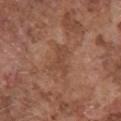Q: Was this lesion biopsied?
A: no biopsy performed (imaged during a skin exam)
Q: How large is the lesion?
A: about 3.5 mm
Q: What kind of image is this?
A: ~15 mm crop, total-body skin-cancer survey
Q: Patient demographics?
A: male, aged 73–77
Q: Where on the body is the lesion?
A: the front of the torso
Q: How was the tile lit?
A: white-light
Q: Automated lesion metrics?
A: a lesion area of about 6 mm², an eccentricity of roughly 0.85, and a shape-asymmetry score of about 0.5 (0 = symmetric); an average lesion color of about L≈45 a*≈22 b*≈30 (CIELAB) and a normalized border contrast of about 5; border irregularity of about 5.5 on a 0–10 scale and radial color variation of about 0.5